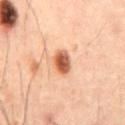Captured during whole-body skin photography for melanoma surveillance; the lesion was not biopsied.
The subject is a male aged approximately 60.
Imaged with cross-polarized lighting.
A lesion tile, about 15 mm wide, cut from a 3D total-body photograph.
The lesion is located on the abdomen.
About 3 mm across.